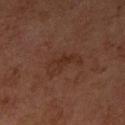workup: catalogued during a skin exam; not biopsied | image source: total-body-photography crop, ~15 mm field of view | location: the right upper arm | patient: female, roughly 60 years of age.